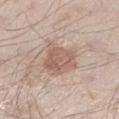Recorded during total-body skin imaging; not selected for excision or biopsy. Automated tile analysis of the lesion measured an area of roughly 12 mm², a shape eccentricity near 0.4, and two-axis asymmetry of about 0.25. The software also gave a mean CIELAB color near L≈59 a*≈18 b*≈25, roughly 10 lightness units darker than nearby skin, and a normalized lesion–skin contrast near 6.5. And it measured a nevus-likeness score of about 60/100. Cropped from a total-body skin-imaging series; the visible field is about 15 mm. This is a white-light tile. A male subject, in their 60s. Located on the left lower leg.